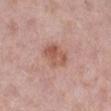Findings:
• follow-up · catalogued during a skin exam; not biopsied
• body site · the right lower leg
• lesion size · ≈4 mm
• image source · ~15 mm tile from a whole-body skin photo
• subject · female, roughly 50 years of age
• tile lighting · white-light